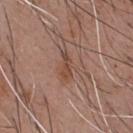Recorded during total-body skin imaging; not selected for excision or biopsy. Located on the chest. The patient is a male aged 53 to 57. A 15 mm crop from a total-body photograph taken for skin-cancer surveillance. The lesion's longest dimension is about 3.5 mm. This is a white-light tile. Automated tile analysis of the lesion measured a shape eccentricity near 0.9 and a shape-asymmetry score of about 0.35 (0 = symmetric). And it measured a lesion color around L≈46 a*≈20 b*≈27 in CIELAB, a lesion–skin lightness drop of about 8, and a normalized border contrast of about 6.5. The software also gave an automated nevus-likeness rating near 5 out of 100 and a detector confidence of about 100 out of 100 that the crop contains a lesion.